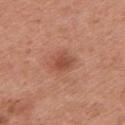Findings:
– notes: imaged on a skin check; not biopsied
– location: the left upper arm
– illumination: white-light
– acquisition: ~15 mm crop, total-body skin-cancer survey
– patient: female, about 50 years old
– diameter: ≈3 mm